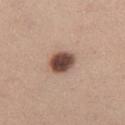The lesion was tiled from a total-body skin photograph and was not biopsied. Imaged with white-light lighting. About 3.5 mm across. A roughly 15 mm field-of-view crop from a total-body skin photograph. A female subject, in their mid-40s. Located on the leg.